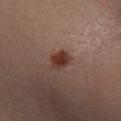Clinical summary:
The recorded lesion diameter is about 3 mm. The total-body-photography lesion software estimated about 11 CIELAB-L* units darker than the surrounding skin and a normalized border contrast of about 10.5. A female patient roughly 40 years of age. A roughly 15 mm field-of-view crop from a total-body skin photograph. Imaged with cross-polarized lighting. On the left leg.